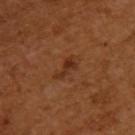| feature | finding |
|---|---|
| biopsy status | total-body-photography surveillance lesion; no biopsy |
| anatomic site | the upper back |
| subject | female, approximately 55 years of age |
| imaging modality | ~15 mm tile from a whole-body skin photo |